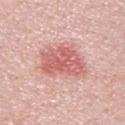notes: total-body-photography surveillance lesion; no biopsy
size: ≈6.5 mm
subject: male, aged around 30
image: total-body-photography crop, ~15 mm field of view
lighting: white-light